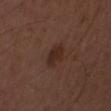Findings:
- follow-up — imaged on a skin check; not biopsied
- size — ≈3 mm
- image source — ~15 mm tile from a whole-body skin photo
- illumination — white-light illumination
- patient — male, roughly 50 years of age